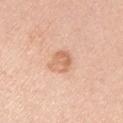Notes:
• workup — imaged on a skin check; not biopsied
• lighting — white-light
• patient — male, in their 40s
• body site — the left upper arm
• acquisition — total-body-photography crop, ~15 mm field of view
• diameter — ≈3 mm
• automated metrics — an eccentricity of roughly 0.6 and a shape-asymmetry score of about 0.3 (0 = symmetric); a classifier nevus-likeness of about 10/100 and lesion-presence confidence of about 100/100